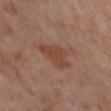  biopsy_status: not biopsied; imaged during a skin examination
  lesion_size:
    long_diameter_mm_approx: 4.0
  automated_metrics:
    cielab_L: 44
    cielab_a: 21
    cielab_b: 29
    vs_skin_darker_L: 7.0
    vs_skin_contrast_norm: 7.0
    nevus_likeness_0_100: 35
    lesion_detection_confidence_0_100: 100
  patient:
    sex: female
    age_approx: 55
  image:
    source: total-body photography crop
    field_of_view_mm: 15
  lighting: cross-polarized
  site: left lower leg